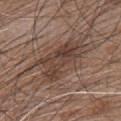| field | value |
|---|---|
| follow-up | imaged on a skin check; not biopsied |
| image source | ~15 mm crop, total-body skin-cancer survey |
| patient | male, aged 43–47 |
| location | the chest |